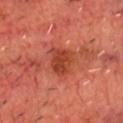Clinical impression:
Imaged during a routine full-body skin examination; the lesion was not biopsied and no histopathology is available.
Clinical summary:
The lesion's longest dimension is about 3.5 mm. A male patient in their 70s. The tile uses cross-polarized illumination. Located on the chest. A lesion tile, about 15 mm wide, cut from a 3D total-body photograph.